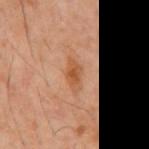{
  "biopsy_status": "not biopsied; imaged during a skin examination",
  "site": "back",
  "patient": {
    "sex": "male",
    "age_approx": 60
  },
  "image": {
    "source": "total-body photography crop",
    "field_of_view_mm": 15
  }
}